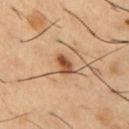follow-up: catalogued during a skin exam; not biopsied
patient: male, roughly 55 years of age
location: the chest
size: ≈2.5 mm
acquisition: 15 mm crop, total-body photography